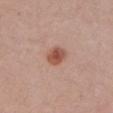The lesion was photographed on a routine skin check and not biopsied; there is no pathology result. This is a white-light tile. Longest diameter approximately 2.5 mm. A male subject approximately 40 years of age. A 15 mm crop from a total-body photograph taken for skin-cancer surveillance. From the left upper arm.